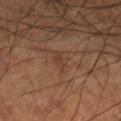Imaged during a routine full-body skin examination; the lesion was not biopsied and no histopathology is available.
From the right lower leg.
The tile uses cross-polarized illumination.
The recorded lesion diameter is about 3 mm.
A male patient aged 58–62.
A 15 mm close-up extracted from a 3D total-body photography capture.